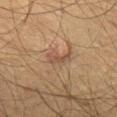Q: Was a biopsy performed?
A: no biopsy performed (imaged during a skin exam)
Q: Lesion location?
A: the left thigh
Q: Lesion size?
A: about 3 mm
Q: What are the patient's age and sex?
A: male, in their mid-60s
Q: Automated lesion metrics?
A: a lesion color around L≈41 a*≈16 b*≈26 in CIELAB, roughly 7 lightness units darker than nearby skin, and a normalized border contrast of about 6; an automated nevus-likeness rating near 10 out of 100 and a lesion-detection confidence of about 90/100
Q: How was this image acquired?
A: ~15 mm tile from a whole-body skin photo
Q: Illumination type?
A: cross-polarized